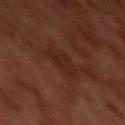Notes:
• lesion diameter: ≈3.5 mm
• lighting: cross-polarized illumination
• subject: male, aged approximately 30
• image: ~15 mm tile from a whole-body skin photo
• location: the left upper arm